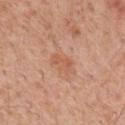| key | value |
|---|---|
| illumination | white-light |
| site | the mid back |
| image-analysis metrics | a lesion area of about 2.5 mm², an eccentricity of roughly 0.9, and two-axis asymmetry of about 0.4; a border-irregularity index near 5/10, internal color variation of about 0 on a 0–10 scale, and peripheral color asymmetry of about 0 |
| acquisition | ~15 mm crop, total-body skin-cancer survey |
| patient | male, in their 60s |